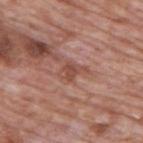Findings:
* notes · catalogued during a skin exam; not biopsied
* body site · the upper back
* image source · ~15 mm crop, total-body skin-cancer survey
* patient · male, in their 70s
* automated lesion analysis · a mean CIELAB color near L≈49 a*≈24 b*≈27 and a normalized lesion–skin contrast near 6.5; border irregularity of about 4 on a 0–10 scale, a color-variation rating of about 2/10, and a peripheral color-asymmetry measure near 0.5